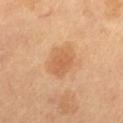Assessment: The lesion was tiled from a total-body skin photograph and was not biopsied. Clinical summary: A male patient, aged 58 to 62. A 15 mm close-up extracted from a 3D total-body photography capture. The lesion is located on the leg.